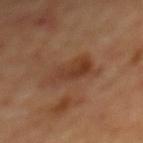* workup — no biopsy performed (imaged during a skin exam)
* patient — male, about 70 years old
* location — the mid back
* automated metrics — a symmetry-axis asymmetry near 0.35; a mean CIELAB color near L≈37 a*≈20 b*≈29, roughly 7 lightness units darker than nearby skin, and a normalized border contrast of about 6.5
* illumination — cross-polarized
* lesion diameter — about 5.5 mm
* imaging modality — total-body-photography crop, ~15 mm field of view